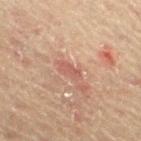follow-up: total-body-photography surveillance lesion; no biopsy | subject: male, in their mid- to late 60s | lighting: cross-polarized illumination | automated metrics: an area of roughly 3 mm² and a shape-asymmetry score of about 0.4 (0 = symmetric); a mean CIELAB color near L≈51 a*≈23 b*≈25 and a normalized border contrast of about 5.5 | lesion size: about 3 mm | anatomic site: the right thigh | imaging modality: ~15 mm tile from a whole-body skin photo.